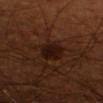Q: How was this image acquired?
A: 15 mm crop, total-body photography
Q: What is the lesion's diameter?
A: ~3.5 mm (longest diameter)
Q: What is the anatomic site?
A: the right upper arm
Q: Who is the patient?
A: male, aged around 70
Q: What lighting was used for the tile?
A: cross-polarized